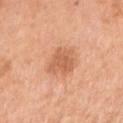The lesion was photographed on a routine skin check and not biopsied; there is no pathology result. Imaged with white-light lighting. The lesion is on the right upper arm. Cropped from a total-body skin-imaging series; the visible field is about 15 mm. The patient is a female in their mid-50s.